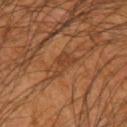Q: Who is the patient?
A: male, aged approximately 60
Q: Lesion location?
A: the left forearm
Q: How was the tile lit?
A: cross-polarized illumination
Q: What did automated image analysis measure?
A: an eccentricity of roughly 0.85
Q: How was this image acquired?
A: total-body-photography crop, ~15 mm field of view